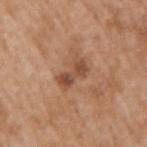Assessment: Recorded during total-body skin imaging; not selected for excision or biopsy. Image and clinical context: The lesion is located on the right upper arm. About 4 mm across. A male subject roughly 65 years of age. A 15 mm close-up tile from a total-body photography series done for melanoma screening. Automated tile analysis of the lesion measured an area of roughly 6.5 mm². It also reported an average lesion color of about L≈49 a*≈22 b*≈32 (CIELAB) and a lesion–skin lightness drop of about 10. The analysis additionally found a color-variation rating of about 4.5/10 and radial color variation of about 1.5.